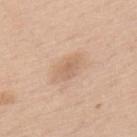Q: Was this lesion biopsied?
A: total-body-photography surveillance lesion; no biopsy
Q: Patient demographics?
A: male, roughly 60 years of age
Q: How large is the lesion?
A: ~3.5 mm (longest diameter)
Q: What kind of image is this?
A: total-body-photography crop, ~15 mm field of view
Q: Lesion location?
A: the upper back
Q: How was the tile lit?
A: white-light